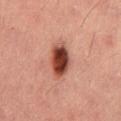| field | value |
|---|---|
| biopsy status | no biopsy performed (imaged during a skin exam) |
| automated metrics | a lesion area of about 10 mm², an eccentricity of roughly 0.75, and a symmetry-axis asymmetry near 0.2; lesion-presence confidence of about 100/100 |
| tile lighting | cross-polarized |
| patient | male, in their mid-50s |
| image source | 15 mm crop, total-body photography |
| anatomic site | the abdomen |